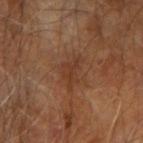Impression: Imaged during a routine full-body skin examination; the lesion was not biopsied and no histopathology is available. Clinical summary: This is a cross-polarized tile. The lesion's longest dimension is about 3.5 mm. A 15 mm close-up extracted from a 3D total-body photography capture. From the right upper arm. The total-body-photography lesion software estimated an area of roughly 5 mm², an eccentricity of roughly 0.8, and two-axis asymmetry of about 0.5. The software also gave an average lesion color of about L≈36 a*≈21 b*≈30 (CIELAB) and a lesion–skin lightness drop of about 6. A male patient, aged around 70.